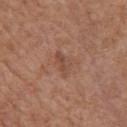Image and clinical context: The patient is a male aged approximately 65. A close-up tile cropped from a whole-body skin photograph, about 15 mm across. On the chest.